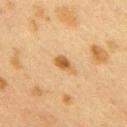follow-up=imaged on a skin check; not biopsied
lighting=cross-polarized
size=~2.5 mm (longest diameter)
subject=female, in their 40s
imaging modality=total-body-photography crop, ~15 mm field of view
location=the right upper arm
automated metrics=an area of roughly 3.5 mm², an eccentricity of roughly 0.8, and a symmetry-axis asymmetry near 0.3; radial color variation of about 1